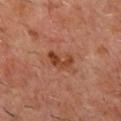Assessment:
This lesion was catalogued during total-body skin photography and was not selected for biopsy.
Background:
Approximately 3 mm at its widest. The patient is a male in their 60s. Located on the front of the torso. Cropped from a whole-body photographic skin survey; the tile spans about 15 mm. This is a cross-polarized tile. The lesion-visualizer software estimated an eccentricity of roughly 0.75 and a symmetry-axis asymmetry near 0.35. And it measured about 9 CIELAB-L* units darker than the surrounding skin and a lesion-to-skin contrast of about 8 (normalized; higher = more distinct). It also reported a border-irregularity index near 3.5/10, a color-variation rating of about 5/10, and a peripheral color-asymmetry measure near 2. And it measured a classifier nevus-likeness of about 35/100.